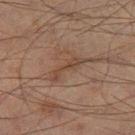Notes:
– automated lesion analysis: a footprint of about 4 mm², an eccentricity of roughly 0.95, and two-axis asymmetry of about 0.6; border irregularity of about 8 on a 0–10 scale, internal color variation of about 0.5 on a 0–10 scale, and a peripheral color-asymmetry measure near 0; a detector confidence of about 80 out of 100 that the crop contains a lesion
– imaging modality: ~15 mm crop, total-body skin-cancer survey
– patient: male, aged approximately 70
– lesion size: about 4.5 mm
– body site: the right thigh
– lighting: cross-polarized